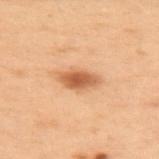| field | value |
|---|---|
| workup | catalogued during a skin exam; not biopsied |
| image source | ~15 mm crop, total-body skin-cancer survey |
| tile lighting | cross-polarized illumination |
| site | the back |
| patient | female, aged around 55 |
| TBP lesion metrics | a lesion color around L≈50 a*≈21 b*≈34 in CIELAB, about 12 CIELAB-L* units darker than the surrounding skin, and a normalized border contrast of about 8.5; border irregularity of about 2.5 on a 0–10 scale, a within-lesion color-variation index near 3.5/10, and peripheral color asymmetry of about 1 |